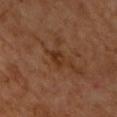Assessment: This lesion was catalogued during total-body skin photography and was not selected for biopsy.